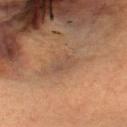Captured during whole-body skin photography for melanoma surveillance; the lesion was not biopsied. A female patient aged 58–62. From the head or neck. A 15 mm close-up tile from a total-body photography series done for melanoma screening.